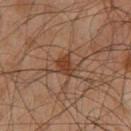Captured during whole-body skin photography for melanoma surveillance; the lesion was not biopsied. Cropped from a total-body skin-imaging series; the visible field is about 15 mm. The subject is a male aged approximately 60. Located on the chest. Approximately 2.5 mm at its widest. Imaged with cross-polarized lighting.